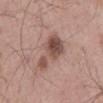biopsy status = imaged on a skin check; not biopsied
subject = male, in their mid- to late 50s
location = the mid back
acquisition = 15 mm crop, total-body photography